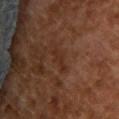{
  "biopsy_status": "not biopsied; imaged during a skin examination",
  "patient": {
    "sex": "female",
    "age_approx": 60
  },
  "image": {
    "source": "total-body photography crop",
    "field_of_view_mm": 15
  },
  "site": "upper back"
}